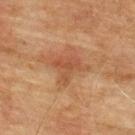Recorded during total-body skin imaging; not selected for excision or biopsy. Cropped from a total-body skin-imaging series; the visible field is about 15 mm. Automated image analysis of the tile measured an area of roughly 7 mm² and an outline eccentricity of about 0.3 (0 = round, 1 = elongated). It also reported a lesion color around L≈42 a*≈20 b*≈30 in CIELAB, a lesion–skin lightness drop of about 7, and a normalized border contrast of about 5.5. The software also gave a within-lesion color-variation index near 2.5/10 and peripheral color asymmetry of about 1. From the upper back. A male subject, aged 73–77. The tile uses cross-polarized illumination.